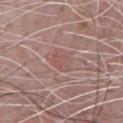Clinical summary: A 15 mm close-up tile from a total-body photography series done for melanoma screening. The recorded lesion diameter is about 2.5 mm. A male patient, in their 70s. Located on the chest.